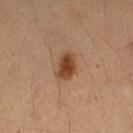<tbp_lesion>
<biopsy_status>not biopsied; imaged during a skin examination</biopsy_status>
<lighting>cross-polarized</lighting>
<site>right forearm</site>
<automated_metrics>
  <area_mm2_approx>6.5</area_mm2_approx>
  <eccentricity>0.75</eccentricity>
  <cielab_L>44</cielab_L>
  <cielab_a>22</cielab_a>
  <cielab_b>33</cielab_b>
  <vs_skin_darker_L>13.0</vs_skin_darker_L>
  <vs_skin_contrast_norm>10.0</vs_skin_contrast_norm>
  <border_irregularity_0_10>2.0</border_irregularity_0_10>
  <color_variation_0_10>3.5</color_variation_0_10>
  <peripheral_color_asymmetry>1.0</peripheral_color_asymmetry>
  <nevus_likeness_0_100>100</nevus_likeness_0_100>
  <lesion_detection_confidence_0_100>100</lesion_detection_confidence_0_100>
</automated_metrics>
<image>
  <source>total-body photography crop</source>
  <field_of_view_mm>15</field_of_view_mm>
</image>
<patient>
  <sex>female</sex>
  <age_approx>35</age_approx>
</patient>
</tbp_lesion>